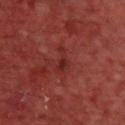The lesion was photographed on a routine skin check and not biopsied; there is no pathology result. This image is a 15 mm lesion crop taken from a total-body photograph. About 3.5 mm across. The lesion is located on the upper back. A male subject, about 70 years old. Captured under cross-polarized illumination.